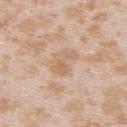{
  "site": "upper back",
  "lighting": "white-light",
  "lesion_size": {
    "long_diameter_mm_approx": 3.0
  },
  "automated_metrics": {
    "cielab_L": 64,
    "cielab_a": 17,
    "cielab_b": 33,
    "vs_skin_darker_L": 7.0,
    "border_irregularity_0_10": 6.0,
    "color_variation_0_10": 1.5,
    "peripheral_color_asymmetry": 0.5
  },
  "patient": {
    "sex": "female",
    "age_approx": 25
  },
  "image": {
    "source": "total-body photography crop",
    "field_of_view_mm": 15
  }
}